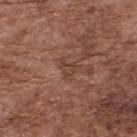Image and clinical context:
This image is a 15 mm lesion crop taken from a total-body photograph. Located on the upper back. The lesion-visualizer software estimated a lesion color around L≈42 a*≈21 b*≈27 in CIELAB. The software also gave a border-irregularity index near 5/10 and a color-variation rating of about 1/10. The analysis additionally found an automated nevus-likeness rating near 0 out of 100 and a lesion-detection confidence of about 85/100. Imaged with white-light lighting. A male subject roughly 75 years of age.